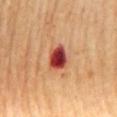No biopsy was performed on this lesion — it was imaged during a full skin examination and was not determined to be concerning.
A female subject, aged 63–67.
The lesion-visualizer software estimated a footprint of about 7 mm², an eccentricity of roughly 0.7, and a shape-asymmetry score of about 0.2 (0 = symmetric). It also reported a mean CIELAB color near L≈44 a*≈35 b*≈31 and a normalized lesion–skin contrast near 14.5. The analysis additionally found a border-irregularity rating of about 1.5/10, a color-variation rating of about 7/10, and radial color variation of about 2.
The lesion's longest dimension is about 3.5 mm.
A roughly 15 mm field-of-view crop from a total-body skin photograph.
From the mid back.
This is a cross-polarized tile.